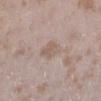Q: How was this image acquired?
A: total-body-photography crop, ~15 mm field of view
Q: Where on the body is the lesion?
A: the right lower leg
Q: What are the patient's age and sex?
A: female, aged 23 to 27